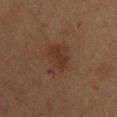<tbp_lesion>
<biopsy_status>not biopsied; imaged during a skin examination</biopsy_status>
<site>upper back</site>
<image>
  <source>total-body photography crop</source>
  <field_of_view_mm>15</field_of_view_mm>
</image>
<patient>
  <sex>male</sex>
  <age_approx>35</age_approx>
</patient>
<lighting>cross-polarized</lighting>
</tbp_lesion>